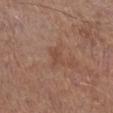Assessment:
The lesion was photographed on a routine skin check and not biopsied; there is no pathology result.
Acquisition and patient details:
Cropped from a total-body skin-imaging series; the visible field is about 15 mm. Approximately 2.5 mm at its widest. On the right lower leg. Imaged with white-light lighting. A male patient in their mid-70s. An algorithmic analysis of the crop reported an area of roughly 3 mm² and a shape-asymmetry score of about 0.35 (0 = symmetric). The software also gave a lesion color around L≈47 a*≈21 b*≈28 in CIELAB, a lesion–skin lightness drop of about 6, and a normalized border contrast of about 5. It also reported a color-variation rating of about 0.5/10. The analysis additionally found a classifier nevus-likeness of about 0/100 and a lesion-detection confidence of about 100/100.